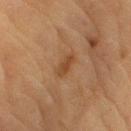Q: Was a biopsy performed?
A: total-body-photography surveillance lesion; no biopsy